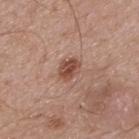Q: Is there a histopathology result?
A: catalogued during a skin exam; not biopsied
Q: What did automated image analysis measure?
A: an automated nevus-likeness rating near 90 out of 100
Q: Where on the body is the lesion?
A: the upper back
Q: How was the tile lit?
A: white-light
Q: Who is the patient?
A: male, aged 68 to 72
Q: What kind of image is this?
A: total-body-photography crop, ~15 mm field of view